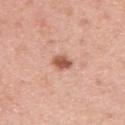notes: imaged on a skin check; not biopsied
site: the arm
image source: 15 mm crop, total-body photography
lighting: white-light
image-analysis metrics: about 15 CIELAB-L* units darker than the surrounding skin; a border-irregularity index near 1.5/10, a color-variation rating of about 2.5/10, and a peripheral color-asymmetry measure near 1
subject: female, in their mid- to late 30s
lesion size: about 2.5 mm